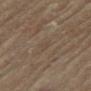Notes:
- follow-up · total-body-photography surveillance lesion; no biopsy
- TBP lesion metrics · an average lesion color of about L≈39 a*≈11 b*≈22 (CIELAB), roughly 3 lightness units darker than nearby skin, and a normalized border contrast of about 3
- anatomic site · the back
- lighting · cross-polarized illumination
- diameter · about 2 mm
- image source · total-body-photography crop, ~15 mm field of view
- patient · female, aged 78–82